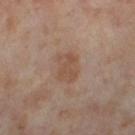| key | value |
|---|---|
| subject | female, approximately 55 years of age |
| anatomic site | the left thigh |
| image source | ~15 mm tile from a whole-body skin photo |
| image-analysis metrics | an area of roughly 7.5 mm², an outline eccentricity of about 0.75 (0 = round, 1 = elongated), and two-axis asymmetry of about 0.25; a lesion color around L≈51 a*≈17 b*≈28 in CIELAB, about 7 CIELAB-L* units darker than the surrounding skin, and a normalized border contrast of about 6 |
| tile lighting | cross-polarized |
| diameter | ~3.5 mm (longest diameter) |